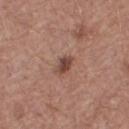follow-up — no biopsy performed (imaged during a skin exam)
acquisition — ~15 mm crop, total-body skin-cancer survey
subject — female, aged around 55
anatomic site — the right thigh
tile lighting — white-light illumination
size — about 2.5 mm
image-analysis metrics — a footprint of about 3 mm² and an eccentricity of roughly 0.75; a lesion color around L≈44 a*≈21 b*≈25 in CIELAB, roughly 12 lightness units darker than nearby skin, and a normalized border contrast of about 9; a border-irregularity rating of about 2/10 and peripheral color asymmetry of about 0.5